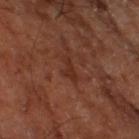{"biopsy_status": "not biopsied; imaged during a skin examination"}